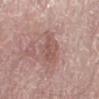Case summary:
• follow-up — catalogued during a skin exam; not biopsied
• image source — total-body-photography crop, ~15 mm field of view
• subject — male, in their 80s
• location — the leg
• lesion diameter — about 4 mm